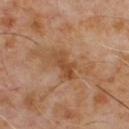Q: Was a biopsy performed?
A: total-body-photography surveillance lesion; no biopsy